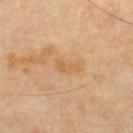Case summary:
* notes · total-body-photography surveillance lesion; no biopsy
* body site · the upper back
* subject · male, roughly 65 years of age
* imaging modality · total-body-photography crop, ~15 mm field of view